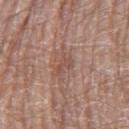Q: Is there a histopathology result?
A: no biopsy performed (imaged during a skin exam)
Q: What is the lesion's diameter?
A: ≈2.5 mm
Q: How was the tile lit?
A: white-light illumination
Q: Lesion location?
A: the leg
Q: What are the patient's age and sex?
A: male, in their 80s
Q: What did automated image analysis measure?
A: a lesion area of about 3.5 mm², an outline eccentricity of about 0.75 (0 = round, 1 = elongated), and two-axis asymmetry of about 0.45; a border-irregularity rating of about 5/10, a within-lesion color-variation index near 1/10, and peripheral color asymmetry of about 0.5
Q: What kind of image is this?
A: ~15 mm crop, total-body skin-cancer survey